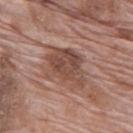{"biopsy_status": "not biopsied; imaged during a skin examination", "patient": {"sex": "male", "age_approx": 70}, "lighting": "white-light", "image": {"source": "total-body photography crop", "field_of_view_mm": 15}, "site": "back", "lesion_size": {"long_diameter_mm_approx": 5.0}}